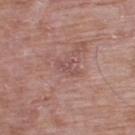{"biopsy_status": "not biopsied; imaged during a skin examination", "site": "upper back", "patient": {"sex": "male", "age_approx": 65}, "image": {"source": "total-body photography crop", "field_of_view_mm": 15}}